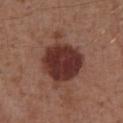- notes: catalogued during a skin exam; not biopsied
- subject: male, in their mid-50s
- acquisition: ~15 mm tile from a whole-body skin photo
- lighting: white-light illumination
- location: the abdomen
- size: ~6 mm (longest diameter)